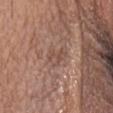No biopsy was performed on this lesion — it was imaged during a full skin examination and was not determined to be concerning.
Automated image analysis of the tile measured a footprint of about 4.5 mm², an eccentricity of roughly 0.65, and a symmetry-axis asymmetry near 0.45. And it measured a lesion color around L≈50 a*≈19 b*≈26 in CIELAB, roughly 7 lightness units darker than nearby skin, and a normalized lesion–skin contrast near 5.5. The software also gave a classifier nevus-likeness of about 0/100 and a detector confidence of about 80 out of 100 that the crop contains a lesion.
The recorded lesion diameter is about 3 mm.
A female subject, aged approximately 50.
On the head or neck.
A close-up tile cropped from a whole-body skin photograph, about 15 mm across.
Imaged with white-light lighting.